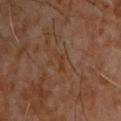Captured during whole-body skin photography for melanoma surveillance; the lesion was not biopsied.
A lesion tile, about 15 mm wide, cut from a 3D total-body photograph.
A male patient in their 60s.
From the chest.
Imaged with cross-polarized lighting.